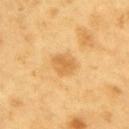workup: imaged on a skin check; not biopsied | automated metrics: a lesion area of about 6.5 mm², an eccentricity of roughly 0.65, and a shape-asymmetry score of about 0.2 (0 = symmetric); a mean CIELAB color near L≈65 a*≈20 b*≈47, a lesion–skin lightness drop of about 9, and a normalized border contrast of about 6 | illumination: cross-polarized | site: the arm | lesion diameter: ≈3.5 mm | patient: male, approximately 60 years of age | image source: 15 mm crop, total-body photography.